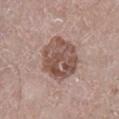Clinical impression:
The lesion was photographed on a routine skin check and not biopsied; there is no pathology result.
Clinical summary:
A region of skin cropped from a whole-body photographic capture, roughly 15 mm wide. Approximately 5.5 mm at its widest. The patient is a male roughly 65 years of age. The lesion is on the right lower leg.